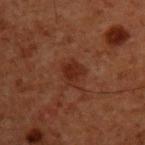notes: no biopsy performed (imaged during a skin exam)
tile lighting: cross-polarized
image: ~15 mm tile from a whole-body skin photo
subject: male, roughly 60 years of age
location: the upper back